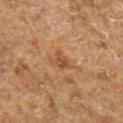Q: Is there a histopathology result?
A: catalogued during a skin exam; not biopsied
Q: What are the patient's age and sex?
A: male, aged 63 to 67
Q: Automated lesion metrics?
A: an area of roughly 4 mm², a shape eccentricity near 0.75, and a symmetry-axis asymmetry near 0.4; a lesion–skin lightness drop of about 8 and a normalized lesion–skin contrast near 6.5
Q: What is the imaging modality?
A: ~15 mm tile from a whole-body skin photo
Q: How was the tile lit?
A: cross-polarized
Q: What is the anatomic site?
A: the left forearm
Q: Lesion size?
A: ≈3 mm